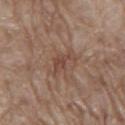Part of a total-body skin-imaging series; this lesion was reviewed on a skin check and was not flagged for biopsy.
A lesion tile, about 15 mm wide, cut from a 3D total-body photograph.
The recorded lesion diameter is about 4 mm.
A male subject in their 70s.
The lesion is located on the mid back.